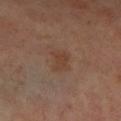<tbp_lesion>
<biopsy_status>not biopsied; imaged during a skin examination</biopsy_status>
<image>
  <source>total-body photography crop</source>
  <field_of_view_mm>15</field_of_view_mm>
</image>
<lighting>cross-polarized</lighting>
<patient>
  <sex>female</sex>
  <age_approx>60</age_approx>
</patient>
<site>left leg</site>
<lesion_size>
  <long_diameter_mm_approx>3.0</long_diameter_mm_approx>
</lesion_size>
</tbp_lesion>